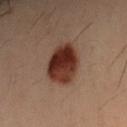Impression:
Recorded during total-body skin imaging; not selected for excision or biopsy.
Context:
A 15 mm crop from a total-body photograph taken for skin-cancer surveillance. About 5.5 mm across. On the left forearm. This is a cross-polarized tile. The total-body-photography lesion software estimated an area of roughly 15 mm², an outline eccentricity of about 0.8 (0 = round, 1 = elongated), and two-axis asymmetry of about 0.15. The analysis additionally found a border-irregularity index near 1.5/10 and a within-lesion color-variation index near 6.5/10. The analysis additionally found an automated nevus-likeness rating near 100 out of 100 and a detector confidence of about 100 out of 100 that the crop contains a lesion. The patient is a male in their 40s.